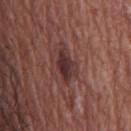Clinical impression: Recorded during total-body skin imaging; not selected for excision or biopsy. Background: Imaged with white-light lighting. The lesion-visualizer software estimated a lesion area of about 7.5 mm², a shape eccentricity near 0.85, and a shape-asymmetry score of about 0.35 (0 = symmetric). And it measured an average lesion color of about L≈33 a*≈21 b*≈19 (CIELAB), a lesion–skin lightness drop of about 10, and a lesion-to-skin contrast of about 9.5 (normalized; higher = more distinct). A male subject aged 73 to 77. The lesion is located on the chest. A lesion tile, about 15 mm wide, cut from a 3D total-body photograph. The lesion's longest dimension is about 4.5 mm.